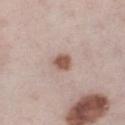notes=imaged on a skin check; not biopsied
subject=male, aged 58–62
imaging modality=15 mm crop, total-body photography
site=the right lower leg
lesion size=~2.5 mm (longest diameter)